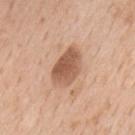No biopsy was performed on this lesion — it was imaged during a full skin examination and was not determined to be concerning.
Captured under white-light illumination.
The lesion is located on the mid back.
A male patient approximately 65 years of age.
A lesion tile, about 15 mm wide, cut from a 3D total-body photograph.
The lesion-visualizer software estimated a border-irregularity rating of about 1.5/10, internal color variation of about 4 on a 0–10 scale, and radial color variation of about 1.5.